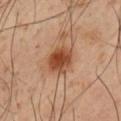notes: catalogued during a skin exam; not biopsied
lighting: cross-polarized illumination
automated metrics: a lesion area of about 9.5 mm², an outline eccentricity of about 0.4 (0 = round, 1 = elongated), and a symmetry-axis asymmetry near 0.15; a lesion color around L≈45 a*≈23 b*≈32 in CIELAB, roughly 13 lightness units darker than nearby skin, and a lesion-to-skin contrast of about 10 (normalized; higher = more distinct); a color-variation rating of about 6.5/10 and peripheral color asymmetry of about 2; an automated nevus-likeness rating near 95 out of 100
image source: ~15 mm crop, total-body skin-cancer survey
site: the left upper arm
subject: male, aged around 50
diameter: ~3.5 mm (longest diameter)